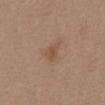{
  "biopsy_status": "not biopsied; imaged during a skin examination",
  "image": {
    "source": "total-body photography crop",
    "field_of_view_mm": 15
  },
  "patient": {
    "sex": "female",
    "age_approx": 40
  },
  "lighting": "white-light",
  "site": "front of the torso",
  "automated_metrics": {
    "area_mm2_approx": 4.0,
    "eccentricity": 0.65,
    "shape_asymmetry": 0.4,
    "color_variation_0_10": 1.5,
    "peripheral_color_asymmetry": 0.5
  }
}